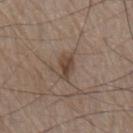follow-up: imaged on a skin check; not biopsied | image-analysis metrics: a lesion color around L≈43 a*≈15 b*≈25 in CIELAB, a lesion–skin lightness drop of about 9, and a normalized border contrast of about 8; an automated nevus-likeness rating near 60 out of 100 and a detector confidence of about 100 out of 100 that the crop contains a lesion | body site: the chest | lighting: white-light illumination | patient: male, roughly 80 years of age | diameter: ~3 mm (longest diameter) | image source: ~15 mm tile from a whole-body skin photo.